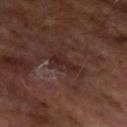No biopsy was performed on this lesion — it was imaged during a full skin examination and was not determined to be concerning. This is a cross-polarized tile. On the left upper arm. A male subject, aged 63–67. A lesion tile, about 15 mm wide, cut from a 3D total-body photograph.